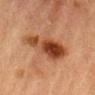Assessment:
This lesion was catalogued during total-body skin photography and was not selected for biopsy.
Clinical summary:
Cropped from a whole-body photographic skin survey; the tile spans about 15 mm. A male subject in their mid-80s or older. From the leg.